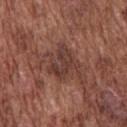workup: catalogued during a skin exam; not biopsied | lighting: white-light | site: the upper back | image source: ~15 mm tile from a whole-body skin photo | patient: male, aged around 75.